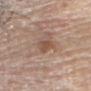Notes:
– notes — catalogued during a skin exam; not biopsied
– image-analysis metrics — an area of roughly 4 mm², a shape eccentricity near 0.7, and a symmetry-axis asymmetry near 0.3; an automated nevus-likeness rating near 5 out of 100 and a detector confidence of about 100 out of 100 that the crop contains a lesion
– subject — male, in their 80s
– imaging modality — total-body-photography crop, ~15 mm field of view
– location — the head or neck
– lesion size — about 2.5 mm